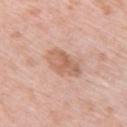Background: Captured under white-light illumination. A lesion tile, about 15 mm wide, cut from a 3D total-body photograph. On the back. A female patient roughly 65 years of age. The lesion-visualizer software estimated an average lesion color of about L≈62 a*≈21 b*≈31 (CIELAB), roughly 10 lightness units darker than nearby skin, and a lesion-to-skin contrast of about 6.5 (normalized; higher = more distinct). The analysis additionally found a border-irregularity rating of about 2.5/10, a color-variation rating of about 4/10, and peripheral color asymmetry of about 1.5. The recorded lesion diameter is about 5 mm.